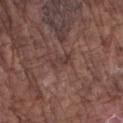This lesion was catalogued during total-body skin photography and was not selected for biopsy.
A male subject aged 73 to 77.
Imaged with white-light lighting.
A region of skin cropped from a whole-body photographic capture, roughly 15 mm wide.
The lesion's longest dimension is about 2.5 mm.
On the arm.